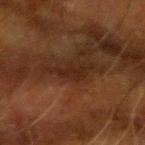Q: Is there a histopathology result?
A: imaged on a skin check; not biopsied
Q: What is the imaging modality?
A: ~15 mm tile from a whole-body skin photo
Q: What is the anatomic site?
A: the left forearm
Q: What are the patient's age and sex?
A: male, in their mid- to late 60s
Q: Lesion size?
A: ≈3 mm